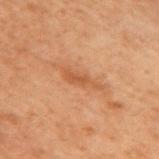Q: Was a biopsy performed?
A: no biopsy performed (imaged during a skin exam)
Q: How large is the lesion?
A: ≈4 mm
Q: Patient demographics?
A: male, about 70 years old
Q: What kind of image is this?
A: ~15 mm tile from a whole-body skin photo
Q: How was the tile lit?
A: cross-polarized
Q: What is the anatomic site?
A: the mid back